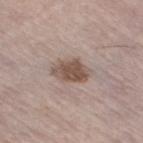Image and clinical context:
The lesion is on the left leg. A lesion tile, about 15 mm wide, cut from a 3D total-body photograph. The subject is a female about 65 years old. About 4 mm across.